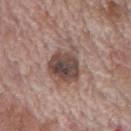  biopsy_status: not biopsied; imaged during a skin examination
  patient:
    sex: male
    age_approx: 70
  image:
    source: total-body photography crop
    field_of_view_mm: 15
  lighting: white-light
  site: mid back
  lesion_size:
    long_diameter_mm_approx: 6.0